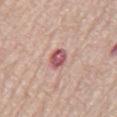Imaged during a routine full-body skin examination; the lesion was not biopsied and no histopathology is available. A male subject, approximately 80 years of age. The lesion's longest dimension is about 3.5 mm. On the chest. The total-body-photography lesion software estimated a shape eccentricity near 0.85 and a symmetry-axis asymmetry near 0.2. And it measured an automated nevus-likeness rating near 0 out of 100 and lesion-presence confidence of about 100/100. Cropped from a whole-body photographic skin survey; the tile spans about 15 mm.